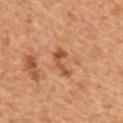Clinical impression:
Imaged during a routine full-body skin examination; the lesion was not biopsied and no histopathology is available.
Acquisition and patient details:
On the arm. A close-up tile cropped from a whole-body skin photograph, about 15 mm across. A male patient roughly 55 years of age. Automated image analysis of the tile measured a footprint of about 4.5 mm², a shape eccentricity near 0.9, and two-axis asymmetry of about 0.5. It also reported an automated nevus-likeness rating near 0 out of 100 and a detector confidence of about 100 out of 100 that the crop contains a lesion.